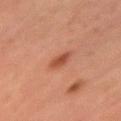This lesion was catalogued during total-body skin photography and was not selected for biopsy.
The recorded lesion diameter is about 2.5 mm.
Automated tile analysis of the lesion measured a footprint of about 4 mm² and an outline eccentricity of about 0.65 (0 = round, 1 = elongated). The software also gave a border-irregularity rating of about 2/10, internal color variation of about 2 on a 0–10 scale, and radial color variation of about 0.5. It also reported an automated nevus-likeness rating near 95 out of 100 and lesion-presence confidence of about 100/100.
A female subject, aged approximately 60.
The lesion is located on the left upper arm.
Imaged with cross-polarized lighting.
A 15 mm close-up tile from a total-body photography series done for melanoma screening.